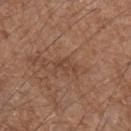| key | value |
|---|---|
| workup | total-body-photography surveillance lesion; no biopsy |
| illumination | white-light illumination |
| image source | ~15 mm tile from a whole-body skin photo |
| image-analysis metrics | a border-irregularity rating of about 5/10, a color-variation rating of about 1.5/10, and radial color variation of about 0.5 |
| anatomic site | the left forearm |
| lesion diameter | ~2.5 mm (longest diameter) |
| patient | male, approximately 55 years of age |